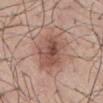{"biopsy_status": "not biopsied; imaged during a skin examination", "image": {"source": "total-body photography crop", "field_of_view_mm": 15}, "lighting": "white-light", "site": "mid back", "lesion_size": {"long_diameter_mm_approx": 8.0}, "automated_metrics": {"area_mm2_approx": 20.0, "eccentricity": 0.9, "shape_asymmetry": 0.2, "cielab_L": 53, "cielab_a": 20, "cielab_b": 26, "vs_skin_darker_L": 11.0, "vs_skin_contrast_norm": 7.5, "nevus_likeness_0_100": 70, "lesion_detection_confidence_0_100": 100}, "patient": {"sex": "male", "age_approx": 55}}